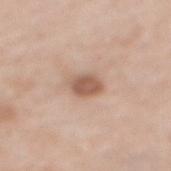Case summary:
- lesion diameter: ≈3 mm
- subject: female, aged approximately 40
- imaging modality: 15 mm crop, total-body photography
- tile lighting: white-light illumination
- image-analysis metrics: a footprint of about 5.5 mm², an eccentricity of roughly 0.6, and a symmetry-axis asymmetry near 0.2; a mean CIELAB color near L≈57 a*≈19 b*≈29, a lesion–skin lightness drop of about 12, and a lesion-to-skin contrast of about 8 (normalized; higher = more distinct); an automated nevus-likeness rating near 80 out of 100 and a detector confidence of about 100 out of 100 that the crop contains a lesion
- anatomic site: the back